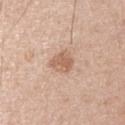{"site": "arm", "lighting": "white-light", "image": {"source": "total-body photography crop", "field_of_view_mm": 15}, "patient": {"sex": "male", "age_approx": 45}}